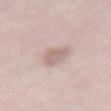Assessment:
Imaged during a routine full-body skin examination; the lesion was not biopsied and no histopathology is available.
Clinical summary:
A female subject in their mid- to late 60s. Measured at roughly 3.5 mm in maximum diameter. The lesion-visualizer software estimated a mean CIELAB color near L≈64 a*≈16 b*≈22, a lesion–skin lightness drop of about 10, and a normalized border contrast of about 6. The lesion is located on the lower back. A lesion tile, about 15 mm wide, cut from a 3D total-body photograph. Imaged with white-light lighting.